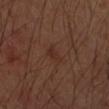Recorded during total-body skin imaging; not selected for excision or biopsy.
Imaged with cross-polarized lighting.
Automated image analysis of the tile measured a border-irregularity index near 4/10, a within-lesion color-variation index near 3/10, and radial color variation of about 1.
Longest diameter approximately 2.5 mm.
A close-up tile cropped from a whole-body skin photograph, about 15 mm across.
The lesion is on the left forearm.